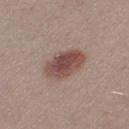Assessment:
This lesion was catalogued during total-body skin photography and was not selected for biopsy.
Acquisition and patient details:
From the left thigh. Measured at roughly 5.5 mm in maximum diameter. The patient is a female about 25 years old. A 15 mm close-up tile from a total-body photography series done for melanoma screening.